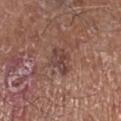notes = imaged on a skin check; not biopsied
acquisition = ~15 mm tile from a whole-body skin photo
illumination = white-light illumination
body site = the leg
patient = male, aged 68 to 72
size = about 3 mm
automated metrics = an automated nevus-likeness rating near 0 out of 100 and lesion-presence confidence of about 100/100